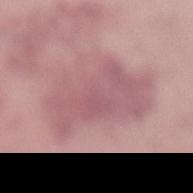Assessment: This lesion was catalogued during total-body skin photography and was not selected for biopsy. Background: A female subject, aged 23–27. Located on the left lower leg. Cropped from a total-body skin-imaging series; the visible field is about 15 mm. Imaged with white-light lighting.